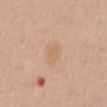Q: Was this lesion biopsied?
A: no biopsy performed (imaged during a skin exam)
Q: What are the patient's age and sex?
A: male, roughly 65 years of age
Q: Lesion location?
A: the front of the torso
Q: Lesion size?
A: ≈3 mm
Q: What is the imaging modality?
A: ~15 mm tile from a whole-body skin photo
Q: Illumination type?
A: white-light